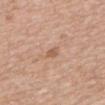Imaged during a routine full-body skin examination; the lesion was not biopsied and no histopathology is available. A male subject, aged approximately 80. Located on the back. The total-body-photography lesion software estimated a footprint of about 2.5 mm², an eccentricity of roughly 0.85, and a symmetry-axis asymmetry near 0.3. A 15 mm close-up tile from a total-body photography series done for melanoma screening.